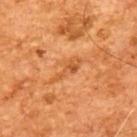biopsy_status: not biopsied; imaged during a skin examination
image:
  source: total-body photography crop
  field_of_view_mm: 15
lighting: cross-polarized
patient:
  sex: male
  age_approx: 65
lesion_size:
  long_diameter_mm_approx: 4.5
automated_metrics:
  cielab_L: 56
  cielab_a: 28
  cielab_b: 45
  vs_skin_darker_L: 8.0
  vs_skin_contrast_norm: 5.5